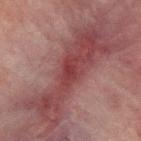Impression: Part of a total-body skin-imaging series; this lesion was reviewed on a skin check and was not flagged for biopsy. Acquisition and patient details: Automated image analysis of the tile measured an eccentricity of roughly 0.85. The software also gave a border-irregularity rating of about 3.5/10, a color-variation rating of about 1.5/10, and radial color variation of about 0.5. And it measured a nevus-likeness score of about 0/100 and a lesion-detection confidence of about 80/100. The patient is a male aged around 70. From the back. Cropped from a whole-body photographic skin survey; the tile spans about 15 mm. The tile uses cross-polarized illumination.